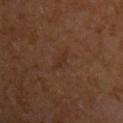Clinical impression:
The lesion was tiled from a total-body skin photograph and was not biopsied.
Context:
A 15 mm close-up tile from a total-body photography series done for melanoma screening. The tile uses cross-polarized illumination. The recorded lesion diameter is about 3 mm. The lesion is on the left upper arm. The patient is a male in their mid-60s.